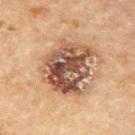Context: The lesion-visualizer software estimated a mean CIELAB color near L≈51 a*≈20 b*≈30, about 17 CIELAB-L* units darker than the surrounding skin, and a normalized border contrast of about 11.5. The analysis additionally found a color-variation rating of about 10/10 and a peripheral color-asymmetry measure near 4. The analysis additionally found an automated nevus-likeness rating near 5 out of 100. Cropped from a whole-body photographic skin survey; the tile spans about 15 mm. Located on the upper back. A male subject, aged 83 or older.